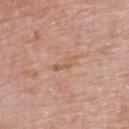Impression: Captured during whole-body skin photography for melanoma surveillance; the lesion was not biopsied. Clinical summary: On the back. The lesion's longest dimension is about 3 mm. A 15 mm close-up tile from a total-body photography series done for melanoma screening. Imaged with white-light lighting. The patient is a male in their mid-70s. The lesion-visualizer software estimated a border-irregularity rating of about 5.5/10, a color-variation rating of about 0/10, and peripheral color asymmetry of about 0.